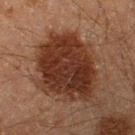Located on the right lower leg.
A male patient, approximately 60 years of age.
Imaged with cross-polarized lighting.
Automated tile analysis of the lesion measured an average lesion color of about L≈24 a*≈18 b*≈22 (CIELAB) and a normalized lesion–skin contrast near 12.
A 15 mm crop from a total-body photograph taken for skin-cancer surveillance.
About 8.5 mm across.